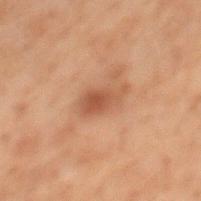Case summary:
- workup — imaged on a skin check; not biopsied
- lighting — cross-polarized
- lesion diameter — about 4 mm
- subject — male, aged 68 to 72
- image-analysis metrics — an automated nevus-likeness rating near 15 out of 100
- body site — the mid back
- image — ~15 mm crop, total-body skin-cancer survey